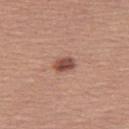Q: Was this lesion biopsied?
A: total-body-photography surveillance lesion; no biopsy
Q: Lesion location?
A: the right thigh
Q: What are the patient's age and sex?
A: female, approximately 50 years of age
Q: How was the tile lit?
A: white-light
Q: Lesion size?
A: ~2.5 mm (longest diameter)
Q: How was this image acquired?
A: 15 mm crop, total-body photography
Q: Automated lesion metrics?
A: an area of roughly 4 mm² and a shape-asymmetry score of about 0.15 (0 = symmetric); a lesion color around L≈49 a*≈23 b*≈27 in CIELAB, about 14 CIELAB-L* units darker than the surrounding skin, and a normalized border contrast of about 9.5; a border-irregularity index near 1.5/10